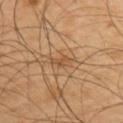No biopsy was performed on this lesion — it was imaged during a full skin examination and was not determined to be concerning. The patient is a male roughly 45 years of age. Imaged with cross-polarized lighting. From the back. A 15 mm close-up extracted from a 3D total-body photography capture. Measured at roughly 3 mm in maximum diameter.